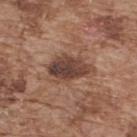No biopsy was performed on this lesion — it was imaged during a full skin examination and was not determined to be concerning. The lesion-visualizer software estimated border irregularity of about 2 on a 0–10 scale, internal color variation of about 6.5 on a 0–10 scale, and peripheral color asymmetry of about 2. The software also gave an automated nevus-likeness rating near 0 out of 100 and a detector confidence of about 100 out of 100 that the crop contains a lesion. Approximately 5 mm at its widest. A male patient, in their mid- to late 70s. This is a white-light tile. From the upper back. Cropped from a total-body skin-imaging series; the visible field is about 15 mm.